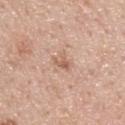A male patient aged around 45. Approximately 2.5 mm at its widest. The lesion is on the upper back. A roughly 15 mm field-of-view crop from a total-body skin photograph. Captured under white-light illumination.